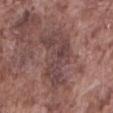follow-up=no biopsy performed (imaged during a skin exam) | image-analysis metrics=a mean CIELAB color near L≈43 a*≈19 b*≈18, about 8 CIELAB-L* units darker than the surrounding skin, and a normalized border contrast of about 7; a border-irregularity rating of about 6.5/10, a within-lesion color-variation index near 4/10, and peripheral color asymmetry of about 1.5; an automated nevus-likeness rating near 0 out of 100 and a lesion-detection confidence of about 60/100 | diameter=~7.5 mm (longest diameter) | image=~15 mm crop, total-body skin-cancer survey | anatomic site=the abdomen | subject=male, aged approximately 75.